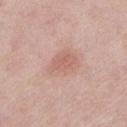biopsy status = total-body-photography surveillance lesion; no biopsy | anatomic site = the left lower leg | image source = ~15 mm tile from a whole-body skin photo | patient = female, aged 58–62.